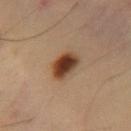Case summary:
• workup · catalogued during a skin exam; not biopsied